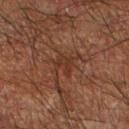biopsy status: total-body-photography surveillance lesion; no biopsy
lighting: cross-polarized illumination
imaging modality: 15 mm crop, total-body photography
automated metrics: a lesion area of about 4.5 mm², an outline eccentricity of about 0.6 (0 = round, 1 = elongated), and a shape-asymmetry score of about 0.4 (0 = symmetric); an average lesion color of about L≈27 a*≈18 b*≈24 (CIELAB), about 5 CIELAB-L* units darker than the surrounding skin, and a normalized lesion–skin contrast near 5.5; a border-irregularity rating of about 4.5/10, a color-variation rating of about 1.5/10, and peripheral color asymmetry of about 0.5; an automated nevus-likeness rating near 0 out of 100 and a lesion-detection confidence of about 70/100
body site: the arm
subject: male, aged 63 to 67